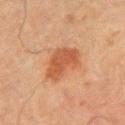{
  "patient": {
    "sex": "male",
    "age_approx": 65
  },
  "site": "left upper arm",
  "image": {
    "source": "total-body photography crop",
    "field_of_view_mm": 15
  }
}